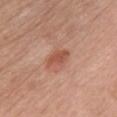notes — no biopsy performed (imaged during a skin exam)
patient — female, aged approximately 55
image source — ~15 mm tile from a whole-body skin photo
site — the chest